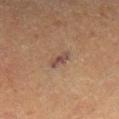Notes:
– follow-up · total-body-photography surveillance lesion; no biopsy
– TBP lesion metrics · an area of roughly 3.5 mm²; a lesion–skin lightness drop of about 8 and a normalized border contrast of about 8; internal color variation of about 0 on a 0–10 scale and radial color variation of about 0
– patient · male, roughly 85 years of age or older
– site · the right lower leg
– tile lighting · cross-polarized
– image · total-body-photography crop, ~15 mm field of view